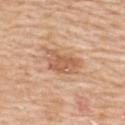Impression:
Imaged during a routine full-body skin examination; the lesion was not biopsied and no histopathology is available.
Background:
The lesion is on the upper back. A male subject about 60 years old. About 5 mm across. This is a white-light tile. Cropped from a total-body skin-imaging series; the visible field is about 15 mm.